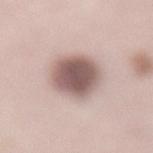This lesion was catalogued during total-body skin photography and was not selected for biopsy. The tile uses white-light illumination. A female subject, approximately 40 years of age. The recorded lesion diameter is about 6 mm. A region of skin cropped from a whole-body photographic capture, roughly 15 mm wide. From the lower back.